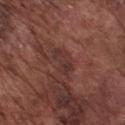Q: Is there a histopathology result?
A: total-body-photography surveillance lesion; no biopsy
Q: How large is the lesion?
A: about 3.5 mm
Q: What is the imaging modality?
A: ~15 mm crop, total-body skin-cancer survey
Q: Illumination type?
A: white-light illumination
Q: Lesion location?
A: the chest
Q: Patient demographics?
A: male, approximately 75 years of age